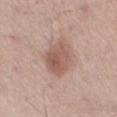This lesion was catalogued during total-body skin photography and was not selected for biopsy. This image is a 15 mm lesion crop taken from a total-body photograph. A male patient in their mid- to late 70s. Imaged with white-light lighting. The lesion is located on the leg.